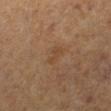Imaged during a routine full-body skin examination; the lesion was not biopsied and no histopathology is available.